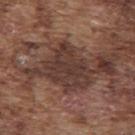notes = catalogued during a skin exam; not biopsied
lighting = white-light
acquisition = ~15 mm tile from a whole-body skin photo
subject = male, in their mid- to late 70s
lesion size = about 5 mm
anatomic site = the upper back